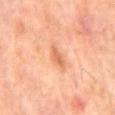follow-up = imaged on a skin check; not biopsied
lesion size = ≈3.5 mm
site = the mid back
imaging modality = total-body-photography crop, ~15 mm field of view
illumination = cross-polarized illumination
patient = male, aged 63–67